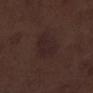biopsy status: catalogued during a skin exam; not biopsied.